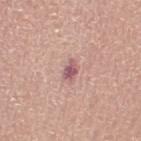The lesion was photographed on a routine skin check and not biopsied; there is no pathology result. Longest diameter approximately 2.5 mm. Located on the leg. The patient is a female aged 63–67. Cropped from a whole-body photographic skin survey; the tile spans about 15 mm. Imaged with white-light lighting.